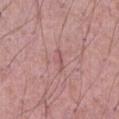notes = imaged on a skin check; not biopsied
image source = total-body-photography crop, ~15 mm field of view
body site = the abdomen
patient = male, in their 70s
lesion size = ~3 mm (longest diameter)